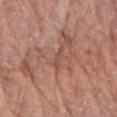Impression: The lesion was photographed on a routine skin check and not biopsied; there is no pathology result. Acquisition and patient details: A region of skin cropped from a whole-body photographic capture, roughly 15 mm wide. A female subject, aged approximately 75. The tile uses white-light illumination. The lesion is on the left forearm. The total-body-photography lesion software estimated a footprint of about 2 mm². The analysis additionally found a lesion color around L≈50 a*≈24 b*≈28 in CIELAB and about 7 CIELAB-L* units darker than the surrounding skin. It also reported a border-irregularity index near 4.5/10, internal color variation of about 0 on a 0–10 scale, and a peripheral color-asymmetry measure near 0. The analysis additionally found an automated nevus-likeness rating near 0 out of 100 and a lesion-detection confidence of about 60/100.